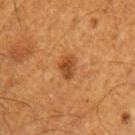workup = total-body-photography surveillance lesion; no biopsy | image = total-body-photography crop, ~15 mm field of view | location = the left upper arm | illumination = cross-polarized | patient = male, aged 58 to 62 | size = ~2.5 mm (longest diameter).